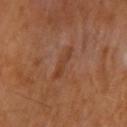| feature | finding |
|---|---|
| workup | imaged on a skin check; not biopsied |
| body site | the left upper arm |
| subject | female, approximately 50 years of age |
| TBP lesion metrics | an average lesion color of about L≈39 a*≈22 b*≈31 (CIELAB), a lesion–skin lightness drop of about 6, and a lesion-to-skin contrast of about 5.5 (normalized; higher = more distinct); a border-irregularity index near 3.5/10, a within-lesion color-variation index near 2.5/10, and radial color variation of about 0.5; an automated nevus-likeness rating near 0 out of 100 and a lesion-detection confidence of about 100/100 |
| lesion diameter | about 3.5 mm |
| imaging modality | ~15 mm crop, total-body skin-cancer survey |